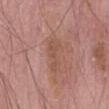notes: imaged on a skin check; not biopsied | patient: male, aged 73–77 | diameter: ~4.5 mm (longest diameter) | site: the front of the torso | image: total-body-photography crop, ~15 mm field of view | image-analysis metrics: an average lesion color of about L≈52 a*≈22 b*≈28 (CIELAB) and a lesion-to-skin contrast of about 5 (normalized; higher = more distinct); internal color variation of about 2 on a 0–10 scale and radial color variation of about 0.5 | tile lighting: white-light illumination.